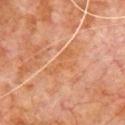  biopsy_status: not biopsied; imaged during a skin examination
  patient:
    sex: male
    age_approx: 80
  image:
    source: total-body photography crop
    field_of_view_mm: 15
  site: chest